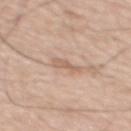{"biopsy_status": "not biopsied; imaged during a skin examination", "lesion_size": {"long_diameter_mm_approx": 3.0}, "image": {"source": "total-body photography crop", "field_of_view_mm": 15}, "lighting": "white-light", "patient": {"sex": "male", "age_approx": 65}, "automated_metrics": {"border_irregularity_0_10": 4.0, "color_variation_0_10": 0.5, "peripheral_color_asymmetry": 0.0}, "site": "mid back"}